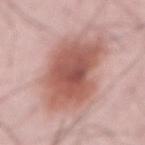Q: Was this lesion biopsied?
A: catalogued during a skin exam; not biopsied
Q: How large is the lesion?
A: ~8 mm (longest diameter)
Q: What lighting was used for the tile?
A: white-light illumination
Q: What are the patient's age and sex?
A: male, in their mid- to late 60s
Q: Where on the body is the lesion?
A: the abdomen
Q: What is the imaging modality?
A: 15 mm crop, total-body photography